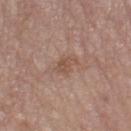Recorded during total-body skin imaging; not selected for excision or biopsy. The patient is a female aged 63 to 67. The recorded lesion diameter is about 2.5 mm. The lesion is located on the left thigh. A 15 mm close-up extracted from a 3D total-body photography capture. An algorithmic analysis of the crop reported an area of roughly 3.5 mm², an outline eccentricity of about 0.85 (0 = round, 1 = elongated), and two-axis asymmetry of about 0.35. And it measured a mean CIELAB color near L≈51 a*≈19 b*≈27, about 7 CIELAB-L* units darker than the surrounding skin, and a normalized border contrast of about 6. The software also gave an automated nevus-likeness rating near 0 out of 100 and a detector confidence of about 100 out of 100 that the crop contains a lesion. This is a white-light tile.